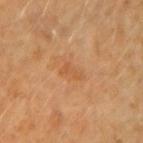Captured during whole-body skin photography for melanoma surveillance; the lesion was not biopsied.